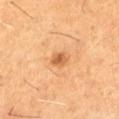Q: Was this lesion biopsied?
A: imaged on a skin check; not biopsied
Q: Lesion location?
A: the right thigh
Q: Automated lesion metrics?
A: a lesion area of about 3.5 mm², a shape eccentricity near 0.4, and a symmetry-axis asymmetry near 0.25; an average lesion color of about L≈58 a*≈25 b*≈41 (CIELAB), a lesion–skin lightness drop of about 11, and a normalized lesion–skin contrast near 7.5
Q: How was this image acquired?
A: ~15 mm tile from a whole-body skin photo
Q: What are the patient's age and sex?
A: male, in their 60s